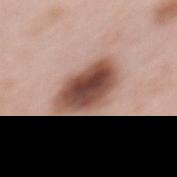  site: mid back
  image:
    source: total-body photography crop
    field_of_view_mm: 15
  patient:
    sex: female
    age_approx: 65